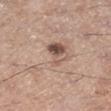Case summary:
– notes: no biopsy performed (imaged during a skin exam)
– subject: male, aged 53–57
– anatomic site: the right lower leg
– imaging modality: ~15 mm tile from a whole-body skin photo